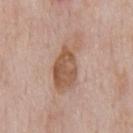Impression: This lesion was catalogued during total-body skin photography and was not selected for biopsy. Image and clinical context: The lesion is on the front of the torso. A 15 mm crop from a total-body photograph taken for skin-cancer surveillance. A male patient roughly 60 years of age. Automated tile analysis of the lesion measured a symmetry-axis asymmetry near 0.35. It also reported an automated nevus-likeness rating near 5 out of 100 and a detector confidence of about 100 out of 100 that the crop contains a lesion. This is a white-light tile.